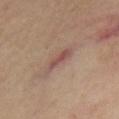Assessment: Recorded during total-body skin imaging; not selected for excision or biopsy. Background: This is a cross-polarized tile. An algorithmic analysis of the crop reported an average lesion color of about L≈51 a*≈21 b*≈23 (CIELAB), roughly 9 lightness units darker than nearby skin, and a normalized lesion–skin contrast near 7. The recorded lesion diameter is about 4 mm. The patient is a female roughly 50 years of age. On the right thigh. A 15 mm close-up extracted from a 3D total-body photography capture.